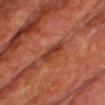Clinical impression:
Imaged during a routine full-body skin examination; the lesion was not biopsied and no histopathology is available.
Clinical summary:
The lesion is on the front of the torso. A 15 mm close-up tile from a total-body photography series done for melanoma screening. The patient is a male roughly 60 years of age.